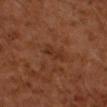Findings:
- follow-up · no biopsy performed (imaged during a skin exam)
- size · ≈3 mm
- patient · male, in their 60s
- location · the right upper arm
- acquisition · ~15 mm crop, total-body skin-cancer survey
- image-analysis metrics · an area of roughly 3 mm², a shape eccentricity near 0.9, and a symmetry-axis asymmetry near 0.4; an automated nevus-likeness rating near 0 out of 100
- lighting · cross-polarized